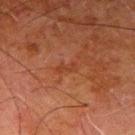Impression: Captured during whole-body skin photography for melanoma surveillance; the lesion was not biopsied. Image and clinical context: Captured under cross-polarized illumination. Located on the arm. A roughly 15 mm field-of-view crop from a total-body skin photograph. The subject is a male aged around 80. Approximately 2.5 mm at its widest.